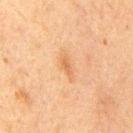Automated tile analysis of the lesion measured an outline eccentricity of about 0.9 (0 = round, 1 = elongated) and a shape-asymmetry score of about 0.4 (0 = symmetric). The software also gave a within-lesion color-variation index near 0/10 and peripheral color asymmetry of about 0. And it measured a nevus-likeness score of about 5/100 and lesion-presence confidence of about 100/100. Located on the chest. A male patient in their mid- to late 70s. About 3 mm across. A region of skin cropped from a whole-body photographic capture, roughly 15 mm wide. The tile uses cross-polarized illumination.